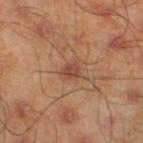Imaged during a routine full-body skin examination; the lesion was not biopsied and no histopathology is available. Approximately 2 mm at its widest. A roughly 15 mm field-of-view crop from a total-body skin photograph. The patient is a male aged around 45. Located on the left lower leg. Automated tile analysis of the lesion measured a footprint of about 3 mm², a shape eccentricity near 0.45, and two-axis asymmetry of about 0.3. The software also gave a lesion color around L≈46 a*≈23 b*≈29 in CIELAB and a lesion-to-skin contrast of about 7 (normalized; higher = more distinct). And it measured a within-lesion color-variation index near 2/10 and radial color variation of about 1.